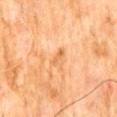<case>
  <biopsy_status>not biopsied; imaged during a skin examination</biopsy_status>
  <automated_metrics>
    <cielab_L>66</cielab_L>
    <cielab_a>25</cielab_a>
    <cielab_b>45</cielab_b>
    <vs_skin_darker_L>8.0</vs_skin_darker_L>
    <vs_skin_contrast_norm>6.0</vs_skin_contrast_norm>
  </automated_metrics>
  <image>
    <source>total-body photography crop</source>
    <field_of_view_mm>15</field_of_view_mm>
  </image>
  <patient>
    <sex>male</sex>
    <age_approx>60</age_approx>
  </patient>
  <lesion_size>
    <long_diameter_mm_approx>2.5</long_diameter_mm_approx>
  </lesion_size>
  <site>abdomen</site>
  <lighting>cross-polarized</lighting>
</case>